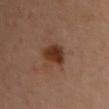Captured during whole-body skin photography for melanoma surveillance; the lesion was not biopsied. The patient is a female in their 40s. About 3.5 mm across. An algorithmic analysis of the crop reported an outline eccentricity of about 0.65 (0 = round, 1 = elongated) and two-axis asymmetry of about 0.2. A region of skin cropped from a whole-body photographic capture, roughly 15 mm wide. Located on the front of the torso. The tile uses cross-polarized illumination.